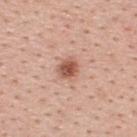Assessment: Imaged during a routine full-body skin examination; the lesion was not biopsied and no histopathology is available. Context: This is a white-light tile. A female patient, in their 40s. On the back. A 15 mm close-up extracted from a 3D total-body photography capture. Longest diameter approximately 2.5 mm.